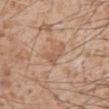Clinical impression: Recorded during total-body skin imaging; not selected for excision or biopsy. Image and clinical context: A 15 mm close-up tile from a total-body photography series done for melanoma screening. The lesion's longest dimension is about 3.5 mm. The tile uses white-light illumination. An algorithmic analysis of the crop reported an outline eccentricity of about 0.85 (0 = round, 1 = elongated) and a symmetry-axis asymmetry near 0.4. The analysis additionally found a nevus-likeness score of about 0/100 and a lesion-detection confidence of about 100/100. A male patient aged 63–67. Located on the abdomen.